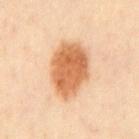notes: catalogued during a skin exam; not biopsied | automated lesion analysis: a shape eccentricity near 0.75 and a shape-asymmetry score of about 0.15 (0 = symmetric); a normalized lesion–skin contrast near 10; a border-irregularity rating of about 1.5/10, internal color variation of about 4 on a 0–10 scale, and radial color variation of about 1.5; a classifier nevus-likeness of about 100/100 and a lesion-detection confidence of about 100/100 | location: the chest | image: total-body-photography crop, ~15 mm field of view | subject: male, approximately 50 years of age | lesion size: ~6.5 mm (longest diameter) | illumination: cross-polarized illumination.